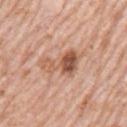{
  "biopsy_status": "not biopsied; imaged during a skin examination",
  "lesion_size": {
    "long_diameter_mm_approx": 5.5
  },
  "lighting": "white-light",
  "site": "left upper arm",
  "automated_metrics": {
    "area_mm2_approx": 10.0,
    "shape_asymmetry": 0.4,
    "cielab_L": 56,
    "cielab_a": 22,
    "cielab_b": 31,
    "vs_skin_contrast_norm": 8.0
  },
  "patient": {
    "sex": "male",
    "age_approx": 80
  },
  "image": {
    "source": "total-body photography crop",
    "field_of_view_mm": 15
  }
}